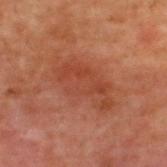Q: Is there a histopathology result?
A: no biopsy performed (imaged during a skin exam)
Q: What are the patient's age and sex?
A: male, aged 68 to 72
Q: How was this image acquired?
A: ~15 mm tile from a whole-body skin photo
Q: Where on the body is the lesion?
A: the upper back